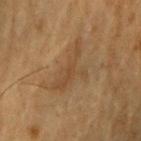| key | value |
|---|---|
| body site | the right upper arm |
| patient | male, in their mid-80s or older |
| image | ~15 mm crop, total-body skin-cancer survey |